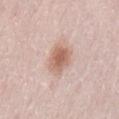Part of a total-body skin-imaging series; this lesion was reviewed on a skin check and was not flagged for biopsy. A female subject aged approximately 30. A 15 mm close-up tile from a total-body photography series done for melanoma screening. The recorded lesion diameter is about 3.5 mm. Imaged with white-light lighting. The lesion is on the lower back.